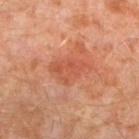Assessment: Captured during whole-body skin photography for melanoma surveillance; the lesion was not biopsied. Clinical summary: Located on the left lower leg. A male subject, roughly 30 years of age. A 15 mm close-up extracted from a 3D total-body photography capture.